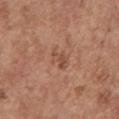Recorded during total-body skin imaging; not selected for excision or biopsy. The patient is a female aged around 65. Approximately 3 mm at its widest. Cropped from a whole-body photographic skin survey; the tile spans about 15 mm. The lesion is located on the right upper arm.